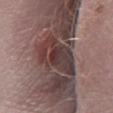This lesion was catalogued during total-body skin photography and was not selected for biopsy. The lesion is on the right lower leg. Imaged with white-light lighting. A male subject approximately 55 years of age. A roughly 15 mm field-of-view crop from a total-body skin photograph.